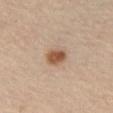<tbp_lesion>
  <biopsy_status>not biopsied; imaged during a skin examination</biopsy_status>
  <patient>
    <sex>male</sex>
    <age_approx>65</age_approx>
  </patient>
  <site>right thigh</site>
  <automated_metrics>
    <border_irregularity_0_10>1.5</border_irregularity_0_10>
    <color_variation_0_10>3.5</color_variation_0_10>
    <peripheral_color_asymmetry>1.5</peripheral_color_asymmetry>
    <nevus_likeness_0_100>100</nevus_likeness_0_100>
    <lesion_detection_confidence_0_100>100</lesion_detection_confidence_0_100>
  </automated_metrics>
  <image>
    <source>total-body photography crop</source>
    <field_of_view_mm>15</field_of_view_mm>
  </image>
</tbp_lesion>